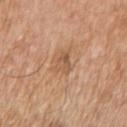Assessment: The lesion was tiled from a total-body skin photograph and was not biopsied. Background: Located on the arm. Imaged with white-light lighting. Measured at roughly 3 mm in maximum diameter. Cropped from a total-body skin-imaging series; the visible field is about 15 mm. The patient is a male aged around 65. The lesion-visualizer software estimated about 9 CIELAB-L* units darker than the surrounding skin and a normalized lesion–skin contrast near 6. The analysis additionally found a border-irregularity index near 4/10 and a color-variation rating of about 3/10. And it measured an automated nevus-likeness rating near 0 out of 100.